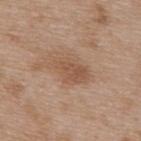{"biopsy_status": "not biopsied; imaged during a skin examination", "automated_metrics": {"area_mm2_approx": 9.5, "eccentricity": 0.8, "shape_asymmetry": 0.3, "cielab_L": 53, "cielab_a": 19, "cielab_b": 30, "vs_skin_darker_L": 8.0, "vs_skin_contrast_norm": 6.0, "color_variation_0_10": 2.5, "peripheral_color_asymmetry": 1.0}, "lesion_size": {"long_diameter_mm_approx": 5.0}, "patient": {"sex": "male", "age_approx": 50}, "site": "upper back", "image": {"source": "total-body photography crop", "field_of_view_mm": 15}, "lighting": "white-light"}